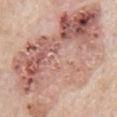biopsy_status: not biopsied; imaged during a skin examination
lighting: white-light
patient:
  sex: male
  age_approx: 65
site: mid back
image:
  source: total-body photography crop
  field_of_view_mm: 15